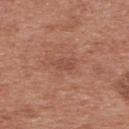Acquisition and patient details:
Imaged with white-light lighting. The lesion-visualizer software estimated a shape eccentricity near 0.8 and two-axis asymmetry of about 0.3. The analysis additionally found a lesion color around L≈49 a*≈24 b*≈29 in CIELAB, roughly 6 lightness units darker than nearby skin, and a normalized lesion–skin contrast near 4.5. The analysis additionally found border irregularity of about 3 on a 0–10 scale and a peripheral color-asymmetry measure near 0.5. On the back. A region of skin cropped from a whole-body photographic capture, roughly 15 mm wide. A male patient aged around 30. Longest diameter approximately 2.5 mm.